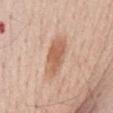Context:
An algorithmic analysis of the crop reported a lesion area of about 10 mm² and a shape eccentricity near 0.85. The software also gave a mean CIELAB color near L≈61 a*≈22 b*≈31, roughly 11 lightness units darker than nearby skin, and a lesion-to-skin contrast of about 7.5 (normalized; higher = more distinct). The software also gave a nevus-likeness score of about 90/100 and a lesion-detection confidence of about 100/100. The patient is a male aged approximately 60. Cropped from a total-body skin-imaging series; the visible field is about 15 mm. Approximately 5 mm at its widest. On the abdomen. This is a white-light tile.